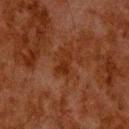Clinical impression: The lesion was photographed on a routine skin check and not biopsied; there is no pathology result. Clinical summary: Approximately 3 mm at its widest. This image is a 15 mm lesion crop taken from a total-body photograph. A male subject roughly 80 years of age. Located on the upper back.